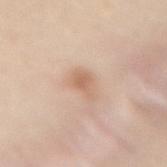{"biopsy_status": "not biopsied; imaged during a skin examination", "automated_metrics": {"area_mm2_approx": 5.5, "eccentricity": 0.75, "shape_asymmetry": 0.35, "cielab_L": 65, "cielab_a": 19, "cielab_b": 31, "vs_skin_darker_L": 9.0, "vs_skin_contrast_norm": 6.0, "border_irregularity_0_10": 3.5, "color_variation_0_10": 3.0, "peripheral_color_asymmetry": 1.0, "nevus_likeness_0_100": 45}, "site": "mid back", "lighting": "white-light", "image": {"source": "total-body photography crop", "field_of_view_mm": 15}, "patient": {"sex": "female", "age_approx": 40}, "lesion_size": {"long_diameter_mm_approx": 3.0}}